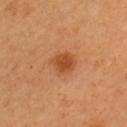workup: catalogued during a skin exam; not biopsied
location: the left upper arm
image source: 15 mm crop, total-body photography
patient: female, approximately 60 years of age